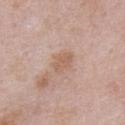Impression:
The lesion was photographed on a routine skin check and not biopsied; there is no pathology result.